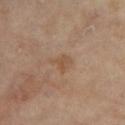<lesion>
<biopsy_status>not biopsied; imaged during a skin examination</biopsy_status>
<image>
  <source>total-body photography crop</source>
  <field_of_view_mm>15</field_of_view_mm>
</image>
<lesion_size>
  <long_diameter_mm_approx>3.0</long_diameter_mm_approx>
</lesion_size>
<site>right lower leg</site>
<patient>
  <sex>female</sex>
  <age_approx>75</age_approx>
</patient>
<lighting>cross-polarized</lighting>
</lesion>